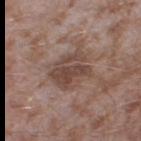Case summary:
* biopsy status: no biopsy performed (imaged during a skin exam)
* anatomic site: the right thigh
* imaging modality: 15 mm crop, total-body photography
* automated lesion analysis: an average lesion color of about L≈45 a*≈17 b*≈23 (CIELAB) and a normalized lesion–skin contrast near 7.5; border irregularity of about 4 on a 0–10 scale, internal color variation of about 4.5 on a 0–10 scale, and radial color variation of about 1.5
* diameter: ~5 mm (longest diameter)
* lighting: white-light illumination
* subject: male, aged 43 to 47